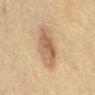Clinical impression: The lesion was photographed on a routine skin check and not biopsied; there is no pathology result.